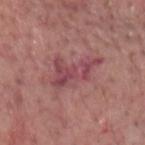Q: Is there a histopathology result?
A: total-body-photography surveillance lesion; no biopsy
Q: Patient demographics?
A: male, aged 78 to 82
Q: What is the imaging modality?
A: ~15 mm tile from a whole-body skin photo
Q: How was the tile lit?
A: white-light illumination
Q: Where on the body is the lesion?
A: the head or neck
Q: What did automated image analysis measure?
A: a mean CIELAB color near L≈47 a*≈28 b*≈18, roughly 9 lightness units darker than nearby skin, and a lesion-to-skin contrast of about 7 (normalized; higher = more distinct)